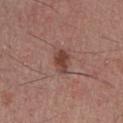workup: catalogued during a skin exam; not biopsied
lesion size: about 3 mm
image: ~15 mm tile from a whole-body skin photo
site: the chest
patient: male, aged approximately 40
lighting: white-light